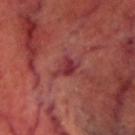Q: Is there a histopathology result?
A: imaged on a skin check; not biopsied
Q: What did automated image analysis measure?
A: a lesion area of about 5 mm² and a shape eccentricity near 0.6; an average lesion color of about L≈35 a*≈32 b*≈20 (CIELAB), roughly 8 lightness units darker than nearby skin, and a normalized lesion–skin contrast near 7.5; a border-irregularity rating of about 4/10, internal color variation of about 3.5 on a 0–10 scale, and a peripheral color-asymmetry measure near 1
Q: Where on the body is the lesion?
A: the head or neck
Q: What are the patient's age and sex?
A: male, aged around 70
Q: How was this image acquired?
A: 15 mm crop, total-body photography
Q: What is the lesion's diameter?
A: ~2.5 mm (longest diameter)
Q: What lighting was used for the tile?
A: cross-polarized